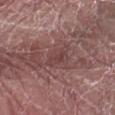This is a white-light tile. A lesion tile, about 15 mm wide, cut from a 3D total-body photograph. The lesion is on the left forearm. Automated tile analysis of the lesion measured a footprint of about 4 mm², an eccentricity of roughly 0.9, and a symmetry-axis asymmetry near 0.6. The analysis additionally found an automated nevus-likeness rating near 0 out of 100. A male patient, aged around 70.